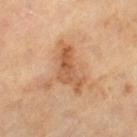Captured during whole-body skin photography for melanoma surveillance; the lesion was not biopsied. Automated image analysis of the tile measured a normalized lesion–skin contrast near 7. And it measured a nevus-likeness score of about 20/100 and lesion-presence confidence of about 100/100. Captured under cross-polarized illumination. A male patient, in their mid-60s. Measured at roughly 6 mm in maximum diameter. A roughly 15 mm field-of-view crop from a total-body skin photograph.